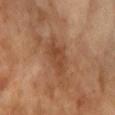Impression: Part of a total-body skin-imaging series; this lesion was reviewed on a skin check and was not flagged for biopsy. Acquisition and patient details: Captured under cross-polarized illumination. A region of skin cropped from a whole-body photographic capture, roughly 15 mm wide. About 3.5 mm across. The lesion is located on the right forearm. Automated tile analysis of the lesion measured a lesion area of about 7 mm². The software also gave a border-irregularity index near 3.5/10 and a peripheral color-asymmetry measure near 1. A female patient, approximately 70 years of age.